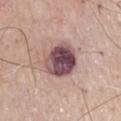body site: the chest
diameter: about 4.5 mm
image source: ~15 mm crop, total-body skin-cancer survey
tile lighting: white-light illumination
image-analysis metrics: a mean CIELAB color near L≈49 a*≈21 b*≈15, a lesion–skin lightness drop of about 19, and a lesion-to-skin contrast of about 13.5 (normalized; higher = more distinct); a border-irregularity index near 1.5/10 and radial color variation of about 3; a nevus-likeness score of about 15/100 and a lesion-detection confidence of about 100/100
patient: male, aged 68 to 72